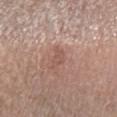| feature | finding |
|---|---|
| workup | no biopsy performed (imaged during a skin exam) |
| site | the left lower leg |
| acquisition | 15 mm crop, total-body photography |
| subject | female, roughly 75 years of age |